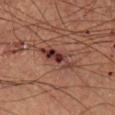{
  "biopsy_status": "not biopsied; imaged during a skin examination",
  "site": "right lower leg",
  "automated_metrics": {
    "area_mm2_approx": 7.0,
    "eccentricity": 0.95,
    "shape_asymmetry": 0.35,
    "border_irregularity_0_10": 5.0,
    "color_variation_0_10": 5.5,
    "peripheral_color_asymmetry": 1.5
  },
  "patient": {
    "sex": "male",
    "age_approx": 55
  },
  "image": {
    "source": "total-body photography crop",
    "field_of_view_mm": 15
  },
  "lighting": "cross-polarized"
}